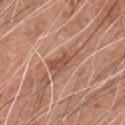No biopsy was performed on this lesion — it was imaged during a full skin examination and was not determined to be concerning. Approximately 4.5 mm at its widest. A 15 mm close-up extracted from a 3D total-body photography capture. Automated image analysis of the tile measured a border-irregularity index near 4/10 and a peripheral color-asymmetry measure near 1. A male patient, approximately 60 years of age. The lesion is on the chest. Captured under white-light illumination.